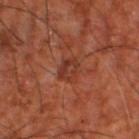follow-up: total-body-photography surveillance lesion; no biopsy
site: the right thigh
lighting: cross-polarized
imaging modality: ~15 mm crop, total-body skin-cancer survey
subject: approximately 65 years of age